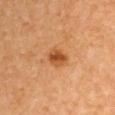Q: Was this lesion biopsied?
A: no biopsy performed (imaged during a skin exam)
Q: Automated lesion metrics?
A: an area of roughly 4.5 mm² and an outline eccentricity of about 0.6 (0 = round, 1 = elongated); a classifier nevus-likeness of about 95/100 and a detector confidence of about 100 out of 100 that the crop contains a lesion
Q: How large is the lesion?
A: ~2.5 mm (longest diameter)
Q: How was the tile lit?
A: cross-polarized illumination
Q: Patient demographics?
A: female, in their mid-50s
Q: What kind of image is this?
A: ~15 mm crop, total-body skin-cancer survey
Q: Where on the body is the lesion?
A: the left upper arm